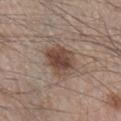This lesion was catalogued during total-body skin photography and was not selected for biopsy.